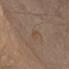This lesion was catalogued during total-body skin photography and was not selected for biopsy. Longest diameter approximately 3 mm. The lesion is located on the chest. This image is a 15 mm lesion crop taken from a total-body photograph. The subject is a male aged 63–67. The lesion-visualizer software estimated an eccentricity of roughly 0.95 and a shape-asymmetry score of about 0.4 (0 = symmetric). The software also gave roughly 5 lightness units darker than nearby skin and a lesion-to-skin contrast of about 5 (normalized; higher = more distinct).